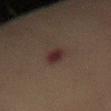biopsy status=catalogued during a skin exam; not biopsied
diameter=~3 mm (longest diameter)
location=the mid back
subject=female, roughly 70 years of age
acquisition=~15 mm crop, total-body skin-cancer survey